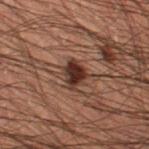Recorded during total-body skin imaging; not selected for excision or biopsy.
Longest diameter approximately 3 mm.
A 15 mm close-up extracted from a 3D total-body photography capture.
This is a cross-polarized tile.
A male subject, aged around 55.
The total-body-photography lesion software estimated a lesion area of about 5.5 mm² and an eccentricity of roughly 0.65. And it measured a border-irregularity index near 2.5/10 and a color-variation rating of about 3/10.
Located on the left thigh.